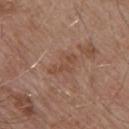Case summary:
• notes — no biopsy performed (imaged during a skin exam)
• lesion size — ~4 mm (longest diameter)
• image source — 15 mm crop, total-body photography
• subject — male, in their mid-50s
• body site — the arm